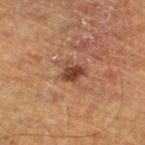Captured during whole-body skin photography for melanoma surveillance; the lesion was not biopsied.
A male patient, approximately 65 years of age.
Located on the left lower leg.
A 15 mm close-up extracted from a 3D total-body photography capture.
The recorded lesion diameter is about 2.5 mm.
Automated tile analysis of the lesion measured a lesion area of about 4.5 mm², a shape eccentricity near 0.6, and two-axis asymmetry of about 0.35. It also reported an average lesion color of about L≈38 a*≈21 b*≈28 (CIELAB) and roughly 11 lightness units darker than nearby skin. The software also gave a border-irregularity rating of about 3/10 and internal color variation of about 4 on a 0–10 scale.